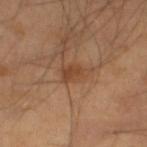This is a cross-polarized tile. A 15 mm close-up extracted from a 3D total-body photography capture. From the left lower leg. About 3 mm across. The patient is a male aged 38 to 42. The total-body-photography lesion software estimated a lesion area of about 5 mm², an eccentricity of roughly 0.8, and two-axis asymmetry of about 0.3. The software also gave a nevus-likeness score of about 15/100.